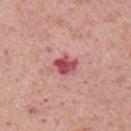Clinical impression:
Part of a total-body skin-imaging series; this lesion was reviewed on a skin check and was not flagged for biopsy.
Context:
A roughly 15 mm field-of-view crop from a total-body skin photograph. Located on the right upper arm. A male patient aged around 70.